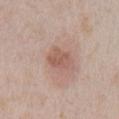– lesion size · ≈3.5 mm
– patient · female, about 60 years old
– anatomic site · the left lower leg
– acquisition · total-body-photography crop, ~15 mm field of view
– tile lighting · white-light